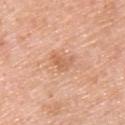No biopsy was performed on this lesion — it was imaged during a full skin examination and was not determined to be concerning.
Imaged with white-light lighting.
The lesion is on the upper back.
The recorded lesion diameter is about 3 mm.
Cropped from a whole-body photographic skin survey; the tile spans about 15 mm.
The lesion-visualizer software estimated a mean CIELAB color near L≈63 a*≈24 b*≈35. The analysis additionally found a nevus-likeness score of about 0/100.
The patient is a male approximately 60 years of age.